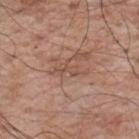Assessment:
Recorded during total-body skin imaging; not selected for excision or biopsy.
Image and clinical context:
A male patient about 70 years old. The lesion-visualizer software estimated an average lesion color of about L≈51 a*≈20 b*≈28 (CIELAB). And it measured an automated nevus-likeness rating near 0 out of 100. This is a white-light tile. Cropped from a total-body skin-imaging series; the visible field is about 15 mm. The lesion is located on the upper back. About 4 mm across.